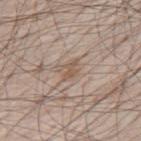Case summary:
* notes · no biopsy performed (imaged during a skin exam)
* site · the mid back
* lesion size · ≈2.5 mm
* image · ~15 mm tile from a whole-body skin photo
* subject · male, aged around 80